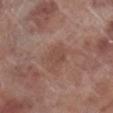Captured during whole-body skin photography for melanoma surveillance; the lesion was not biopsied. The lesion is located on the right lower leg. The lesion's longest dimension is about 2.5 mm. The tile uses white-light illumination. The subject is a male roughly 70 years of age. This image is a 15 mm lesion crop taken from a total-body photograph. The total-body-photography lesion software estimated an average lesion color of about L≈47 a*≈19 b*≈25 (CIELAB) and about 6 CIELAB-L* units darker than the surrounding skin. And it measured border irregularity of about 1.5 on a 0–10 scale.